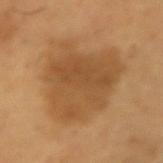workup: imaged on a skin check; not biopsied | TBP lesion metrics: a mean CIELAB color near L≈50 a*≈20 b*≈39, about 9 CIELAB-L* units darker than the surrounding skin, and a lesion-to-skin contrast of about 6.5 (normalized; higher = more distinct); border irregularity of about 4 on a 0–10 scale and a within-lesion color-variation index near 3.5/10; an automated nevus-likeness rating near 55 out of 100 | patient: male, about 65 years old | lesion size: ≈8 mm | tile lighting: cross-polarized illumination | location: the right upper arm | image source: ~15 mm crop, total-body skin-cancer survey.